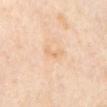notes: no biopsy performed (imaged during a skin exam) | acquisition: 15 mm crop, total-body photography | subject: female, approximately 50 years of age | tile lighting: cross-polarized illumination | TBP lesion metrics: an area of roughly 3 mm² and a symmetry-axis asymmetry near 0.45; a within-lesion color-variation index near 0/10 and radial color variation of about 0; a nevus-likeness score of about 0/100 and a detector confidence of about 100 out of 100 that the crop contains a lesion | site: the abdomen | size: about 3 mm.